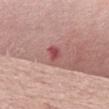The lesion was tiled from a total-body skin photograph and was not biopsied.
A roughly 15 mm field-of-view crop from a total-body skin photograph.
From the front of the torso.
The tile uses white-light illumination.
A female subject, aged 58 to 62.
The lesion's longest dimension is about 2.5 mm.
The total-body-photography lesion software estimated a footprint of about 4.5 mm² and a shape-asymmetry score of about 0.3 (0 = symmetric). The analysis additionally found a within-lesion color-variation index near 5/10 and radial color variation of about 1.5. The analysis additionally found a nevus-likeness score of about 0/100.